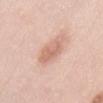biopsy status: catalogued during a skin exam; not biopsied
location: the abdomen
subject: female, aged 28–32
tile lighting: white-light illumination
image: ~15 mm tile from a whole-body skin photo